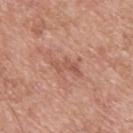Findings:
* biopsy status — no biopsy performed (imaged during a skin exam)
* location — the upper back
* tile lighting — white-light
* lesion diameter — ~4 mm (longest diameter)
* subject — male, about 55 years old
* imaging modality — total-body-photography crop, ~15 mm field of view